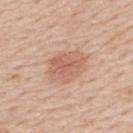workup = total-body-photography surveillance lesion; no biopsy | image = ~15 mm crop, total-body skin-cancer survey | subject = male, about 60 years old | image-analysis metrics = a classifier nevus-likeness of about 90/100 and a lesion-detection confidence of about 100/100 | body site = the mid back | diameter = about 4.5 mm | tile lighting = white-light illumination.